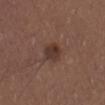biopsy_status: not biopsied; imaged during a skin examination
patient:
  sex: male
  age_approx: 30
lesion_size:
  long_diameter_mm_approx: 3.0
automated_metrics:
  area_mm2_approx: 5.5
  eccentricity: 0.65
  shape_asymmetry: 0.2
  cielab_L: 34
  cielab_a: 18
  cielab_b: 22
  vs_skin_darker_L: 9.0
  nevus_likeness_0_100: 85
  lesion_detection_confidence_0_100: 100
image:
  source: total-body photography crop
  field_of_view_mm: 15
lighting: white-light
site: right upper arm